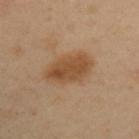patient: male, in their 40s
illumination: cross-polarized illumination
location: the left upper arm
automated metrics: an area of roughly 14 mm²; lesion-presence confidence of about 100/100
imaging modality: 15 mm crop, total-body photography
lesion diameter: ≈5.5 mm